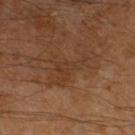patient: male, roughly 60 years of age | illumination: cross-polarized | acquisition: total-body-photography crop, ~15 mm field of view | image-analysis metrics: an area of roughly 11 mm², an eccentricity of roughly 0.85, and a symmetry-axis asymmetry near 0.6; a mean CIELAB color near L≈33 a*≈18 b*≈27, roughly 5 lightness units darker than nearby skin, and a normalized border contrast of about 5; border irregularity of about 8.5 on a 0–10 scale, a within-lesion color-variation index near 2.5/10, and a peripheral color-asymmetry measure near 0.5; a nevus-likeness score of about 0/100 and a lesion-detection confidence of about 95/100 | lesion size: about 6 mm | anatomic site: the left lower leg.